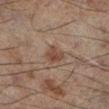Findings:
– acquisition: total-body-photography crop, ~15 mm field of view
– size: ~2.5 mm (longest diameter)
– site: the left lower leg
– patient: male, about 60 years old